The patient is a male in their 20s; this image is a 15 mm lesion crop taken from a total-body photograph; the lesion is on the left upper arm: 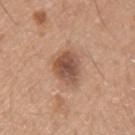Q: How was the tile lit?
A: white-light illumination
Q: What did automated image analysis measure?
A: a lesion–skin lightness drop of about 13 and a normalized border contrast of about 9
Q: Lesion size?
A: about 3.5 mm
Q: What did pathology find?
A: a dysplastic (Clark) nevus